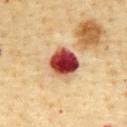<tbp_lesion>
<biopsy_status>not biopsied; imaged during a skin examination</biopsy_status>
<lighting>cross-polarized</lighting>
<site>chest</site>
<image>
  <source>total-body photography crop</source>
  <field_of_view_mm>15</field_of_view_mm>
</image>
<patient>
  <sex>male</sex>
  <age_approx>65</age_approx>
</patient>
<lesion_size>
  <long_diameter_mm_approx>4.0</long_diameter_mm_approx>
</lesion_size>
</tbp_lesion>